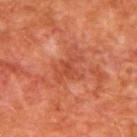notes=catalogued during a skin exam; not biopsied
subject=male, about 65 years old
tile lighting=cross-polarized
body site=the upper back
image source=15 mm crop, total-body photography